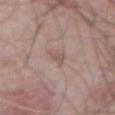notes: catalogued during a skin exam; not biopsied
image: ~15 mm crop, total-body skin-cancer survey
location: the abdomen
subject: male, roughly 70 years of age
automated lesion analysis: an area of roughly 3 mm², a shape eccentricity near 0.9, and a shape-asymmetry score of about 0.4 (0 = symmetric); a mean CIELAB color near L≈54 a*≈17 b*≈22, roughly 7 lightness units darker than nearby skin, and a normalized border contrast of about 5
lighting: white-light illumination
diameter: ≈3.5 mm